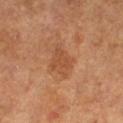Q: Is there a histopathology result?
A: imaged on a skin check; not biopsied
Q: What are the patient's age and sex?
A: female, aged 63 to 67
Q: Lesion size?
A: about 3.5 mm
Q: What lighting was used for the tile?
A: cross-polarized
Q: How was this image acquired?
A: total-body-photography crop, ~15 mm field of view
Q: What is the anatomic site?
A: the left lower leg
Q: What did automated image analysis measure?
A: a footprint of about 7.5 mm², an eccentricity of roughly 0.65, and a shape-asymmetry score of about 0.3 (0 = symmetric)